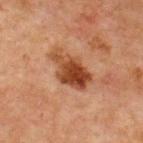Q: Was a biopsy performed?
A: total-body-photography surveillance lesion; no biopsy
Q: Patient demographics?
A: male, aged around 65
Q: Automated lesion metrics?
A: a lesion area of about 12 mm² and an outline eccentricity of about 0.85 (0 = round, 1 = elongated); an automated nevus-likeness rating near 20 out of 100 and a lesion-detection confidence of about 100/100
Q: Where on the body is the lesion?
A: the chest
Q: What is the imaging modality?
A: 15 mm crop, total-body photography
Q: Illumination type?
A: cross-polarized
Q: What is the lesion's diameter?
A: ≈5.5 mm